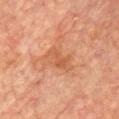biopsy status — no biopsy performed (imaged during a skin exam)
anatomic site — the chest
subject — male, about 75 years old
image source — 15 mm crop, total-body photography
size — about 4 mm